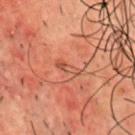Impression:
The lesion was tiled from a total-body skin photograph and was not biopsied.
Image and clinical context:
Longest diameter approximately 3 mm. A region of skin cropped from a whole-body photographic capture, roughly 15 mm wide. From the chest. Captured under cross-polarized illumination. A male patient about 50 years old. The lesion-visualizer software estimated a classifier nevus-likeness of about 0/100 and a detector confidence of about 95 out of 100 that the crop contains a lesion.